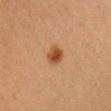* biopsy status — catalogued during a skin exam; not biopsied
* tile lighting — cross-polarized
* acquisition — ~15 mm tile from a whole-body skin photo
* patient — female, approximately 45 years of age
* image-analysis metrics — a border-irregularity index near 1.5/10 and a within-lesion color-variation index near 3.5/10
* anatomic site — the chest
* diameter — about 2.5 mm